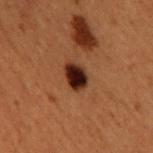Q: Was this lesion biopsied?
A: total-body-photography surveillance lesion; no biopsy
Q: Patient demographics?
A: male, aged around 50
Q: How was the tile lit?
A: cross-polarized illumination
Q: How large is the lesion?
A: about 3 mm
Q: Lesion location?
A: the upper back
Q: What kind of image is this?
A: ~15 mm tile from a whole-body skin photo